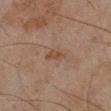Q: Is there a histopathology result?
A: no biopsy performed (imaged during a skin exam)
Q: How large is the lesion?
A: ≈3 mm
Q: Lesion location?
A: the right lower leg
Q: Patient demographics?
A: male, roughly 45 years of age
Q: How was the tile lit?
A: cross-polarized
Q: What is the imaging modality?
A: 15 mm crop, total-body photography